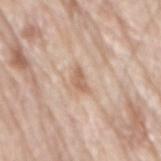{"biopsy_status": "not biopsied; imaged during a skin examination", "site": "mid back", "patient": {"sex": "male", "age_approx": 80}, "image": {"source": "total-body photography crop", "field_of_view_mm": 15}, "lighting": "white-light"}